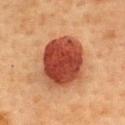Q: Was this lesion biopsied?
A: catalogued during a skin exam; not biopsied
Q: How was the tile lit?
A: cross-polarized
Q: What are the patient's age and sex?
A: female, aged 58 to 62
Q: What is the lesion's diameter?
A: about 6.5 mm
Q: Lesion location?
A: the upper back
Q: How was this image acquired?
A: 15 mm crop, total-body photography